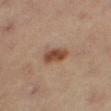Clinical impression: Captured during whole-body skin photography for melanoma surveillance; the lesion was not biopsied. Image and clinical context: Measured at roughly 3.5 mm in maximum diameter. The total-body-photography lesion software estimated a shape eccentricity near 0.8 and a shape-asymmetry score of about 0.25 (0 = symmetric). On the right thigh. The subject is a male aged around 55. A 15 mm close-up tile from a total-body photography series done for melanoma screening.